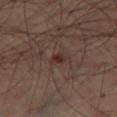<case>
<biopsy_status>not biopsied; imaged during a skin examination</biopsy_status>
<lighting>cross-polarized</lighting>
<automated_metrics>
  <area_mm2_approx>2.0</area_mm2_approx>
  <eccentricity>0.55</eccentricity>
  <shape_asymmetry>0.3</shape_asymmetry>
  <cielab_L>28</cielab_L>
  <cielab_a>16</cielab_a>
  <cielab_b>22</cielab_b>
  <vs_skin_darker_L>8.0</vs_skin_darker_L>
</automated_metrics>
<lesion_size>
  <long_diameter_mm_approx>1.5</long_diameter_mm_approx>
</lesion_size>
<patient>
  <sex>male</sex>
  <age_approx>65</age_approx>
</patient>
<site>right thigh</site>
<image>
  <source>total-body photography crop</source>
  <field_of_view_mm>15</field_of_view_mm>
</image>
</case>